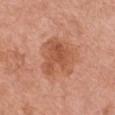Impression:
Part of a total-body skin-imaging series; this lesion was reviewed on a skin check and was not flagged for biopsy.
Clinical summary:
The recorded lesion diameter is about 5 mm. This is a white-light tile. Automated tile analysis of the lesion measured a mean CIELAB color near L≈54 a*≈26 b*≈34 and a lesion–skin lightness drop of about 9. It also reported an automated nevus-likeness rating near 20 out of 100 and a detector confidence of about 100 out of 100 that the crop contains a lesion. A female patient, in their mid-60s. A 15 mm close-up tile from a total-body photography series done for melanoma screening. Located on the chest.